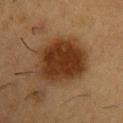Q: Was a biopsy performed?
A: imaged on a skin check; not biopsied
Q: What is the anatomic site?
A: the left upper arm
Q: Who is the patient?
A: male, aged around 55
Q: How large is the lesion?
A: about 7 mm
Q: What kind of image is this?
A: total-body-photography crop, ~15 mm field of view
Q: Illumination type?
A: cross-polarized illumination